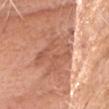Clinical impression: Captured during whole-body skin photography for melanoma surveillance; the lesion was not biopsied. Context: Measured at roughly 5.5 mm in maximum diameter. On the head or neck. A close-up tile cropped from a whole-body skin photograph, about 15 mm across. A female subject, aged 73 to 77.